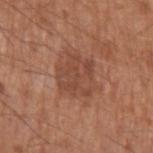The subject is a male about 65 years old. The lesion is on the left upper arm. A close-up tile cropped from a whole-body skin photograph, about 15 mm across.